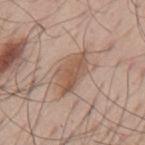| key | value |
|---|---|
| notes | total-body-photography surveillance lesion; no biopsy |
| anatomic site | the mid back |
| imaging modality | ~15 mm crop, total-body skin-cancer survey |
| diameter | about 5.5 mm |
| subject | male, aged 53–57 |
| illumination | white-light illumination |
| image-analysis metrics | an average lesion color of about L≈54 a*≈18 b*≈28 (CIELAB), about 10 CIELAB-L* units darker than the surrounding skin, and a lesion-to-skin contrast of about 7 (normalized; higher = more distinct); a border-irregularity index near 4/10 and internal color variation of about 3.5 on a 0–10 scale; a classifier nevus-likeness of about 60/100 |